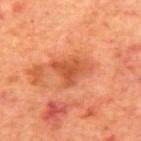Clinical impression:
No biopsy was performed on this lesion — it was imaged during a full skin examination and was not determined to be concerning.